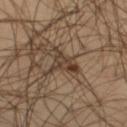follow-up: no biopsy performed (imaged during a skin exam).